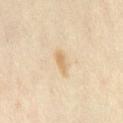| feature | finding |
|---|---|
| biopsy status | total-body-photography surveillance lesion; no biopsy |
| automated lesion analysis | a nevus-likeness score of about 30/100 and a lesion-detection confidence of about 100/100 |
| subject | female, approximately 35 years of age |
| site | the mid back |
| lesion size | about 2.5 mm |
| illumination | cross-polarized illumination |
| acquisition | ~15 mm crop, total-body skin-cancer survey |